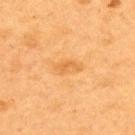Impression: Captured during whole-body skin photography for melanoma surveillance; the lesion was not biopsied. Clinical summary: On the upper back. A female patient, approximately 40 years of age. Automated image analysis of the tile measured a mean CIELAB color near L≈57 a*≈23 b*≈44 and about 7 CIELAB-L* units darker than the surrounding skin. Imaged with cross-polarized lighting. A roughly 15 mm field-of-view crop from a total-body skin photograph. The recorded lesion diameter is about 3 mm.